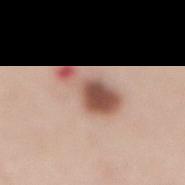workup = total-body-photography surveillance lesion; no biopsy
illumination = white-light
subject = female, aged around 50
size = ~6 mm (longest diameter)
site = the mid back
image = total-body-photography crop, ~15 mm field of view